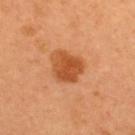lighting — cross-polarized
patient — female, aged 48 to 52
image — ~15 mm tile from a whole-body skin photo
TBP lesion metrics — an average lesion color of about L≈52 a*≈29 b*≈43 (CIELAB), roughly 12 lightness units darker than nearby skin, and a normalized border contrast of about 8.5; a border-irregularity rating of about 2.5/10, internal color variation of about 4 on a 0–10 scale, and a peripheral color-asymmetry measure near 1; a nevus-likeness score of about 95/100 and a detector confidence of about 100 out of 100 that the crop contains a lesion
size — about 4 mm